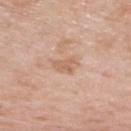{
  "biopsy_status": "not biopsied; imaged during a skin examination",
  "site": "upper back",
  "image": {
    "source": "total-body photography crop",
    "field_of_view_mm": 15
  },
  "patient": {
    "sex": "male",
    "age_approx": 60
  }
}